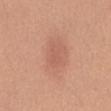notes: catalogued during a skin exam; not biopsied
patient: female, aged approximately 55
size: ~3 mm (longest diameter)
location: the mid back
image source: total-body-photography crop, ~15 mm field of view
lighting: white-light